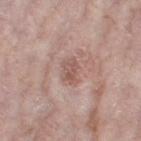notes: no biopsy performed (imaged during a skin exam)
body site: the right thigh
TBP lesion metrics: an outline eccentricity of about 0.8 (0 = round, 1 = elongated); a classifier nevus-likeness of about 0/100 and a lesion-detection confidence of about 100/100
image source: ~15 mm tile from a whole-body skin photo
patient: female, aged 68–72